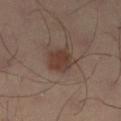notes — catalogued during a skin exam; not biopsied
tile lighting — cross-polarized
anatomic site — the left lower leg
acquisition — 15 mm crop, total-body photography
patient — male, aged approximately 40
diameter — about 3.5 mm